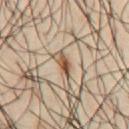workup — no biopsy performed (imaged during a skin exam) | acquisition — total-body-photography crop, ~15 mm field of view | diameter — ≈2.5 mm | subject — male, approximately 40 years of age | TBP lesion metrics — an eccentricity of roughly 0.85 and a shape-asymmetry score of about 0.3 (0 = symmetric); an average lesion color of about L≈49 a*≈18 b*≈32 (CIELAB), about 16 CIELAB-L* units darker than the surrounding skin, and a lesion-to-skin contrast of about 11.5 (normalized; higher = more distinct); border irregularity of about 3 on a 0–10 scale, a color-variation rating of about 2/10, and peripheral color asymmetry of about 0.5 | location — the chest.